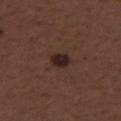Clinical summary:
Measured at roughly 2.5 mm in maximum diameter. Captured under white-light illumination. The patient is a female aged 48–52. On the back. This image is a 15 mm lesion crop taken from a total-body photograph. The lesion-visualizer software estimated a normalized lesion–skin contrast near 10.5. It also reported border irregularity of about 1 on a 0–10 scale, internal color variation of about 3 on a 0–10 scale, and radial color variation of about 1. It also reported an automated nevus-likeness rating near 90 out of 100 and a detector confidence of about 100 out of 100 that the crop contains a lesion.